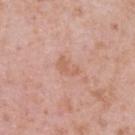Q: Is there a histopathology result?
A: no biopsy performed (imaged during a skin exam)
Q: Lesion size?
A: ~2.5 mm (longest diameter)
Q: What is the anatomic site?
A: the back
Q: How was this image acquired?
A: total-body-photography crop, ~15 mm field of view
Q: What are the patient's age and sex?
A: female, in their 40s
Q: What did automated image analysis measure?
A: a footprint of about 3 mm² and a shape eccentricity near 0.85; a lesion color around L≈61 a*≈23 b*≈31 in CIELAB, roughly 7 lightness units darker than nearby skin, and a lesion-to-skin contrast of about 5.5 (normalized; higher = more distinct); a border-irregularity index near 5/10 and a within-lesion color-variation index near 0.5/10